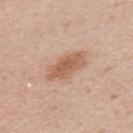follow-up = catalogued during a skin exam; not biopsied | subject = female, aged around 30 | image = ~15 mm crop, total-body skin-cancer survey | lesion diameter = ≈5 mm | location = the upper back | automated metrics = a border-irregularity index near 3/10, a within-lesion color-variation index near 3/10, and peripheral color asymmetry of about 1; an automated nevus-likeness rating near 90 out of 100 and a lesion-detection confidence of about 100/100 | illumination = white-light illumination.